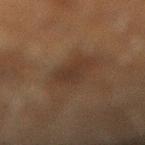Q: Was a biopsy performed?
A: imaged on a skin check; not biopsied
Q: Where on the body is the lesion?
A: the right lower leg
Q: Lesion size?
A: about 3.5 mm
Q: What did automated image analysis measure?
A: a lesion area of about 6.5 mm² and a shape eccentricity near 0.8; an average lesion color of about L≈25 a*≈13 b*≈21 (CIELAB), about 5 CIELAB-L* units darker than the surrounding skin, and a normalized border contrast of about 6; a border-irregularity index near 3.5/10 and a within-lesion color-variation index near 2/10
Q: What are the patient's age and sex?
A: male, in their 60s
Q: What kind of image is this?
A: total-body-photography crop, ~15 mm field of view